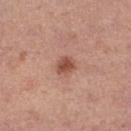The lesion's longest dimension is about 2.5 mm. Cropped from a whole-body photographic skin survey; the tile spans about 15 mm. On the left thigh. A female patient in their 40s.